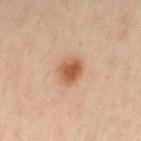Findings:
• biopsy status: total-body-photography surveillance lesion; no biopsy
• image source: 15 mm crop, total-body photography
• location: the leg
• patient: female, in their 40s